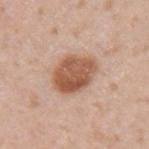Impression:
The lesion was photographed on a routine skin check and not biopsied; there is no pathology result.
Background:
Captured under white-light illumination. The patient is a male aged approximately 35. From the left upper arm. Approximately 5 mm at its widest. The lesion-visualizer software estimated a shape eccentricity near 0.65. And it measured about 14 CIELAB-L* units darker than the surrounding skin and a normalized border contrast of about 9.5. The analysis additionally found a lesion-detection confidence of about 100/100. Cropped from a whole-body photographic skin survey; the tile spans about 15 mm.